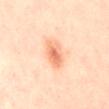Notes:
– follow-up — imaged on a skin check; not biopsied
– image source — ~15 mm tile from a whole-body skin photo
– anatomic site — the lower back
– subject — female, in their mid-40s